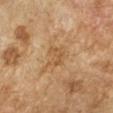patient:
  sex: female
  age_approx: 70
image:
  source: total-body photography crop
  field_of_view_mm: 15
lighting: cross-polarized
site: right forearm